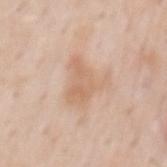biopsy status: catalogued during a skin exam; not biopsied | patient: male, aged approximately 60 | size: ≈5 mm | tile lighting: white-light illumination | automated lesion analysis: a lesion–skin lightness drop of about 7; a border-irregularity rating of about 5.5/10 and a peripheral color-asymmetry measure near 1 | location: the mid back | imaging modality: 15 mm crop, total-body photography.